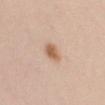Clinical impression: Imaged during a routine full-body skin examination; the lesion was not biopsied and no histopathology is available. Acquisition and patient details: This is a white-light tile. A female subject, about 25 years old. From the chest. A 15 mm close-up extracted from a 3D total-body photography capture. The lesion's longest dimension is about 2.5 mm.